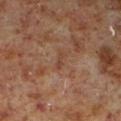| field | value |
|---|---|
| biopsy status | imaged on a skin check; not biopsied |
| subject | male, approximately 60 years of age |
| image source | ~15 mm tile from a whole-body skin photo |
| lighting | cross-polarized illumination |
| site | the left lower leg |